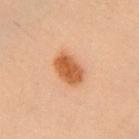No biopsy was performed on this lesion — it was imaged during a full skin examination and was not determined to be concerning.
The total-body-photography lesion software estimated a lesion color around L≈47 a*≈22 b*≈34 in CIELAB, a lesion–skin lightness drop of about 11, and a normalized lesion–skin contrast near 9.5. It also reported a within-lesion color-variation index near 3/10 and a peripheral color-asymmetry measure near 1.
A roughly 15 mm field-of-view crop from a total-body skin photograph.
A female subject approximately 40 years of age.
About 4 mm across.
This is a cross-polarized tile.
Located on the chest.